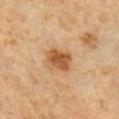{
  "biopsy_status": "not biopsied; imaged during a skin examination",
  "site": "right upper arm",
  "automated_metrics": {
    "cielab_L": 46,
    "cielab_a": 19,
    "cielab_b": 35,
    "vs_skin_darker_L": 11.0,
    "vs_skin_contrast_norm": 8.5,
    "border_irregularity_0_10": 1.5,
    "color_variation_0_10": 4.5,
    "peripheral_color_asymmetry": 1.5,
    "nevus_likeness_0_100": 95
  },
  "lesion_size": {
    "long_diameter_mm_approx": 3.5
  },
  "patient": {
    "sex": "male",
    "age_approx": 65
  },
  "image": {
    "source": "total-body photography crop",
    "field_of_view_mm": 15
  }
}